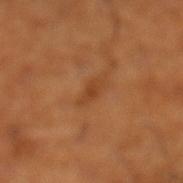patient:
  sex: male
  age_approx: 65
automated_metrics:
  cielab_L: 43
  cielab_a: 25
  cielab_b: 38
  vs_skin_darker_L: 7.0
  vs_skin_contrast_norm: 6.0
  border_irregularity_0_10: 3.5
  color_variation_0_10: 1.0
site: left lower leg
lesion_size:
  long_diameter_mm_approx: 3.0
image:
  source: total-body photography crop
  field_of_view_mm: 15